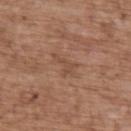Captured during whole-body skin photography for melanoma surveillance; the lesion was not biopsied. A male patient aged 68 to 72. A roughly 15 mm field-of-view crop from a total-body skin photograph. The lesion is located on the upper back.